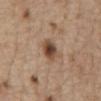Clinical impression:
Recorded during total-body skin imaging; not selected for excision or biopsy.
Clinical summary:
A lesion tile, about 15 mm wide, cut from a 3D total-body photograph. Imaged with white-light lighting. A male patient, aged around 70. Measured at roughly 3 mm in maximum diameter. From the abdomen. An algorithmic analysis of the crop reported a lesion area of about 6 mm², an eccentricity of roughly 0.65, and a symmetry-axis asymmetry near 0.15. And it measured an average lesion color of about L≈46 a*≈18 b*≈28 (CIELAB) and a normalized lesion–skin contrast near 10. The software also gave a border-irregularity index near 1.5/10, internal color variation of about 7 on a 0–10 scale, and a peripheral color-asymmetry measure near 2.